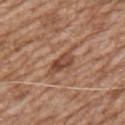Impression:
No biopsy was performed on this lesion — it was imaged during a full skin examination and was not determined to be concerning.
Clinical summary:
A roughly 15 mm field-of-view crop from a total-body skin photograph. The lesion is located on the right upper arm. A male patient approximately 60 years of age.